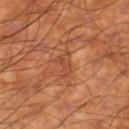Q: Was this lesion biopsied?
A: total-body-photography surveillance lesion; no biopsy
Q: What did automated image analysis measure?
A: an average lesion color of about L≈47 a*≈26 b*≈34 (CIELAB), a lesion–skin lightness drop of about 6, and a normalized border contrast of about 5; a nevus-likeness score of about 0/100 and a detector confidence of about 70 out of 100 that the crop contains a lesion
Q: Patient demographics?
A: male, in their 60s
Q: How was this image acquired?
A: total-body-photography crop, ~15 mm field of view
Q: Illumination type?
A: cross-polarized
Q: What is the anatomic site?
A: the right lower leg